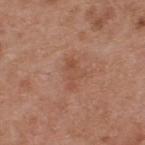Imaged during a routine full-body skin examination; the lesion was not biopsied and no histopathology is available. On the upper back. The patient is a male aged around 55. This is a white-light tile. A roughly 15 mm field-of-view crop from a total-body skin photograph.